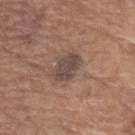Context:
A close-up tile cropped from a whole-body skin photograph, about 15 mm across. Longest diameter approximately 4 mm. The subject is a male aged approximately 80. Located on the left upper arm. Captured under white-light illumination.